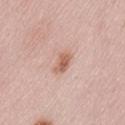Findings:
• notes · catalogued during a skin exam; not biopsied
• location · the lower back
• subject · female, aged 68 to 72
• image-analysis metrics · a footprint of about 4.5 mm², a shape eccentricity near 0.8, and two-axis asymmetry of about 0.25; a mean CIELAB color near L≈62 a*≈21 b*≈29, about 11 CIELAB-L* units darker than the surrounding skin, and a normalized border contrast of about 7.5
• image · 15 mm crop, total-body photography